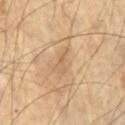{"patient": {"sex": "male", "age_approx": 60}, "image": {"source": "total-body photography crop", "field_of_view_mm": 15}, "site": "right upper arm"}